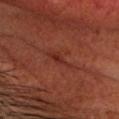Clinical summary:
Imaged with cross-polarized lighting. The lesion is located on the head or neck. A roughly 15 mm field-of-view crop from a total-body skin photograph. The subject is a male in their mid- to late 50s.